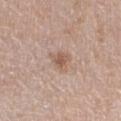Captured during whole-body skin photography for melanoma surveillance; the lesion was not biopsied.
Captured under white-light illumination.
Cropped from a total-body skin-imaging series; the visible field is about 15 mm.
Measured at roughly 3 mm in maximum diameter.
The lesion is on the right thigh.
A female patient aged around 60.